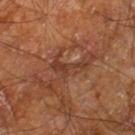follow-up = imaged on a skin check; not biopsied
image-analysis metrics = a lesion color around L≈36 a*≈22 b*≈29 in CIELAB, a lesion–skin lightness drop of about 7, and a normalized border contrast of about 6.5; border irregularity of about 8.5 on a 0–10 scale, a color-variation rating of about 1/10, and radial color variation of about 0
site = the leg
patient = male, approximately 60 years of age
diameter = ~4.5 mm (longest diameter)
imaging modality = ~15 mm tile from a whole-body skin photo